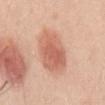Q: Is there a histopathology result?
A: imaged on a skin check; not biopsied
Q: Where on the body is the lesion?
A: the mid back
Q: How was the tile lit?
A: white-light illumination
Q: What is the imaging modality?
A: 15 mm crop, total-body photography
Q: What are the patient's age and sex?
A: male, in their mid-20s